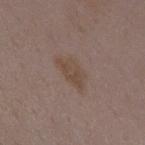Assessment:
Recorded during total-body skin imaging; not selected for excision or biopsy.
Image and clinical context:
The patient is a female in their mid-30s. About 4 mm across. This image is a 15 mm lesion crop taken from a total-body photograph. The lesion is located on the mid back. The lesion-visualizer software estimated a shape eccentricity near 0.9 and two-axis asymmetry of about 0.35. And it measured a border-irregularity rating of about 4/10, internal color variation of about 2 on a 0–10 scale, and radial color variation of about 1.